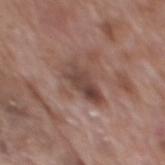notes: no biopsy performed (imaged during a skin exam); location: the mid back; image source: ~15 mm tile from a whole-body skin photo; patient: male, roughly 70 years of age; illumination: white-light illumination; lesion diameter: about 4.5 mm.